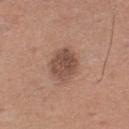{
  "biopsy_status": "not biopsied; imaged during a skin examination",
  "site": "left lower leg",
  "patient": {
    "sex": "male",
    "age_approx": 75
  },
  "image": {
    "source": "total-body photography crop",
    "field_of_view_mm": 15
  }
}